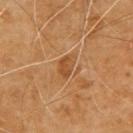This lesion was catalogued during total-body skin photography and was not selected for biopsy. An algorithmic analysis of the crop reported a lesion color around L≈46 a*≈22 b*≈37 in CIELAB, roughly 8 lightness units darker than nearby skin, and a normalized border contrast of about 6. And it measured a border-irregularity rating of about 2/10, a within-lesion color-variation index near 3/10, and a peripheral color-asymmetry measure near 1. This is a cross-polarized tile. A male subject approximately 60 years of age. Approximately 3 mm at its widest. The lesion is located on the upper back. A region of skin cropped from a whole-body photographic capture, roughly 15 mm wide.